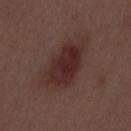Q: Was a biopsy performed?
A: imaged on a skin check; not biopsied